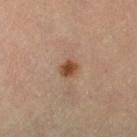Q: Is there a histopathology result?
A: no biopsy performed (imaged during a skin exam)
Q: Automated lesion metrics?
A: a lesion color around L≈44 a*≈20 b*≈31 in CIELAB
Q: Who is the patient?
A: female, aged around 65
Q: Where on the body is the lesion?
A: the left leg
Q: Illumination type?
A: cross-polarized
Q: What is the lesion's diameter?
A: ≈2 mm
Q: How was this image acquired?
A: ~15 mm tile from a whole-body skin photo